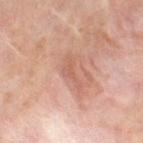The lesion was photographed on a routine skin check and not biopsied; there is no pathology result.
Measured at roughly 4 mm in maximum diameter.
The lesion is located on the leg.
A roughly 15 mm field-of-view crop from a total-body skin photograph.
A female subject, aged 48–52.